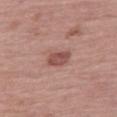Part of a total-body skin-imaging series; this lesion was reviewed on a skin check and was not flagged for biopsy.
The recorded lesion diameter is about 3 mm.
A female subject, aged 63–67.
A region of skin cropped from a whole-body photographic capture, roughly 15 mm wide.
On the right thigh.
This is a white-light tile.
The lesion-visualizer software estimated border irregularity of about 2 on a 0–10 scale, internal color variation of about 2.5 on a 0–10 scale, and radial color variation of about 1. And it measured a classifier nevus-likeness of about 75/100 and a detector confidence of about 100 out of 100 that the crop contains a lesion.